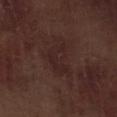This lesion was catalogued during total-body skin photography and was not selected for biopsy. A close-up tile cropped from a whole-body skin photograph, about 15 mm across. The subject is a male roughly 70 years of age. Longest diameter approximately 5 mm. This is a white-light tile. The lesion is on the right lower leg.